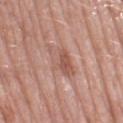| key | value |
|---|---|
| follow-up | catalogued during a skin exam; not biopsied |
| lighting | white-light |
| size | ≈5.5 mm |
| location | the leg |
| subject | female, roughly 70 years of age |
| acquisition | total-body-photography crop, ~15 mm field of view |